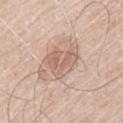The lesion was tiled from a total-body skin photograph and was not biopsied.
This is a white-light tile.
A male subject aged 73 to 77.
Automated image analysis of the tile measured a classifier nevus-likeness of about 5/100 and a lesion-detection confidence of about 100/100.
A close-up tile cropped from a whole-body skin photograph, about 15 mm across.
The lesion's longest dimension is about 4.5 mm.
The lesion is located on the left upper arm.